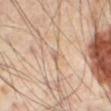notes: imaged on a skin check; not biopsied | anatomic site: the abdomen | subject: male, aged 63 to 67 | size: about 1.5 mm | image source: ~15 mm tile from a whole-body skin photo | tile lighting: cross-polarized illumination | automated metrics: an area of roughly 1 mm², an outline eccentricity of about 0.75 (0 = round, 1 = elongated), and two-axis asymmetry of about 0.35; a mean CIELAB color near L≈58 a*≈17 b*≈27, roughly 8 lightness units darker than nearby skin, and a normalized lesion–skin contrast near 5.5; border irregularity of about 2.5 on a 0–10 scale, internal color variation of about 0 on a 0–10 scale, and radial color variation of about 0.